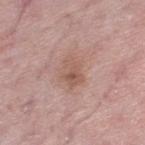Clinical impression: Part of a total-body skin-imaging series; this lesion was reviewed on a skin check and was not flagged for biopsy. Context: The subject is a female in their 60s. Captured under white-light illumination. Cropped from a whole-body photographic skin survey; the tile spans about 15 mm. Approximately 3.5 mm at its widest. The lesion is located on the leg. An algorithmic analysis of the crop reported an area of roughly 6 mm², an outline eccentricity of about 0.75 (0 = round, 1 = elongated), and two-axis asymmetry of about 0.4. The software also gave a border-irregularity rating of about 4/10, a within-lesion color-variation index near 4.5/10, and radial color variation of about 1.5. The software also gave a classifier nevus-likeness of about 0/100.